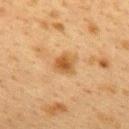Impression: Imaged during a routine full-body skin examination; the lesion was not biopsied and no histopathology is available. Image and clinical context: The lesion is on the upper back. Automated tile analysis of the lesion measured an average lesion color of about L≈47 a*≈19 b*≈37 (CIELAB) and a normalized border contrast of about 8. It also reported a color-variation rating of about 3.5/10. The software also gave a classifier nevus-likeness of about 85/100 and a detector confidence of about 100 out of 100 that the crop contains a lesion. The lesion's longest dimension is about 2.5 mm. A lesion tile, about 15 mm wide, cut from a 3D total-body photograph. The subject is a female in their 40s. Imaged with cross-polarized lighting.